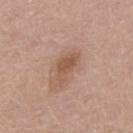{
  "biopsy_status": "not biopsied; imaged during a skin examination",
  "lighting": "white-light",
  "patient": {
    "sex": "male",
    "age_approx": 55
  },
  "image": {
    "source": "total-body photography crop",
    "field_of_view_mm": 15
  },
  "lesion_size": {
    "long_diameter_mm_approx": 4.5
  },
  "site": "chest"
}